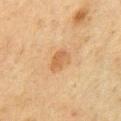{
  "biopsy_status": "not biopsied; imaged during a skin examination",
  "patient": {
    "sex": "male",
    "age_approx": 75
  },
  "automated_metrics": {
    "area_mm2_approx": 5.0,
    "shape_asymmetry": 0.25,
    "cielab_L": 49,
    "cielab_a": 17,
    "cielab_b": 34,
    "vs_skin_darker_L": 7.0,
    "vs_skin_contrast_norm": 6.0,
    "border_irregularity_0_10": 2.5
  },
  "lesion_size": {
    "long_diameter_mm_approx": 3.0
  },
  "image": {
    "source": "total-body photography crop",
    "field_of_view_mm": 15
  },
  "site": "chest",
  "lighting": "cross-polarized"
}